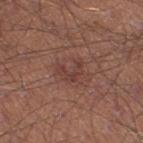Q: Is there a histopathology result?
A: catalogued during a skin exam; not biopsied
Q: What is the imaging modality?
A: ~15 mm tile from a whole-body skin photo
Q: Who is the patient?
A: male, aged around 45
Q: Where on the body is the lesion?
A: the right thigh
Q: Lesion size?
A: ≈3 mm
Q: What lighting was used for the tile?
A: white-light